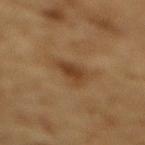Clinical impression:
This lesion was catalogued during total-body skin photography and was not selected for biopsy.
Background:
The recorded lesion diameter is about 3 mm. A 15 mm close-up tile from a total-body photography series done for melanoma screening. The subject is a male approximately 85 years of age or older. An algorithmic analysis of the crop reported an eccentricity of roughly 0.7 and two-axis asymmetry of about 0.35. The analysis additionally found a mean CIELAB color near L≈34 a*≈17 b*≈30 and a lesion–skin lightness drop of about 8. The analysis additionally found a border-irregularity rating of about 3/10 and radial color variation of about 0.5. The analysis additionally found an automated nevus-likeness rating near 75 out of 100. The lesion is located on the mid back. This is a cross-polarized tile.